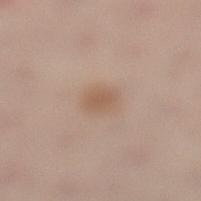This lesion was catalogued during total-body skin photography and was not selected for biopsy.